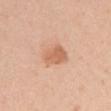Q: Was this lesion biopsied?
A: catalogued during a skin exam; not biopsied
Q: Lesion size?
A: ~3.5 mm (longest diameter)
Q: What did automated image analysis measure?
A: a symmetry-axis asymmetry near 0.2; an average lesion color of about L≈64 a*≈23 b*≈34 (CIELAB), about 10 CIELAB-L* units darker than the surrounding skin, and a lesion-to-skin contrast of about 6.5 (normalized; higher = more distinct); a border-irregularity index near 2/10, a color-variation rating of about 2.5/10, and peripheral color asymmetry of about 1; a classifier nevus-likeness of about 50/100
Q: Who is the patient?
A: female, aged around 35
Q: Where on the body is the lesion?
A: the left upper arm
Q: How was the tile lit?
A: white-light illumination
Q: What kind of image is this?
A: ~15 mm crop, total-body skin-cancer survey